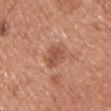| feature | finding |
|---|---|
| notes | total-body-photography surveillance lesion; no biopsy |
| automated lesion analysis | a footprint of about 7.5 mm², a shape eccentricity near 0.4, and a symmetry-axis asymmetry near 0.2; border irregularity of about 2.5 on a 0–10 scale, a within-lesion color-variation index near 3/10, and peripheral color asymmetry of about 1; a classifier nevus-likeness of about 45/100 and a detector confidence of about 100 out of 100 that the crop contains a lesion |
| site | the chest |
| size | ~3.5 mm (longest diameter) |
| subject | female, aged around 35 |
| illumination | white-light illumination |
| image source | 15 mm crop, total-body photography |